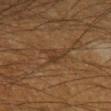follow-up = catalogued during a skin exam; not biopsied.